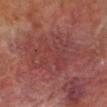Assessment: No biopsy was performed on this lesion — it was imaged during a full skin examination and was not determined to be concerning. Background: The lesion's longest dimension is about 7 mm. A 15 mm crop from a total-body photograph taken for skin-cancer surveillance. The patient is a male approximately 70 years of age. Imaged with cross-polarized lighting. On the right lower leg.